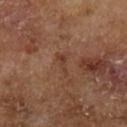– lesion diameter — about 3 mm
– tile lighting — cross-polarized illumination
– subject — male, roughly 65 years of age
– image — total-body-photography crop, ~15 mm field of view